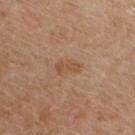Q: Was this lesion biopsied?
A: total-body-photography surveillance lesion; no biopsy
Q: How was this image acquired?
A: total-body-photography crop, ~15 mm field of view
Q: Who is the patient?
A: male, aged around 70
Q: How was the tile lit?
A: white-light illumination
Q: What did automated image analysis measure?
A: a classifier nevus-likeness of about 15/100 and a detector confidence of about 100 out of 100 that the crop contains a lesion
Q: Lesion location?
A: the chest
Q: What is the lesion's diameter?
A: ~3 mm (longest diameter)